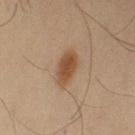Part of a total-body skin-imaging series; this lesion was reviewed on a skin check and was not flagged for biopsy. The tile uses cross-polarized illumination. Cropped from a whole-body photographic skin survey; the tile spans about 15 mm. On the arm. A male patient about 40 years old.